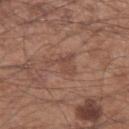Clinical impression:
Recorded during total-body skin imaging; not selected for excision or biopsy.
Context:
Automated tile analysis of the lesion measured roughly 7 lightness units darker than nearby skin and a normalized lesion–skin contrast near 5. The analysis additionally found a nevus-likeness score of about 0/100 and lesion-presence confidence of about 90/100. Imaged with white-light lighting. The lesion is located on the left forearm. A 15 mm close-up extracted from a 3D total-body photography capture. A male subject, aged around 55. Approximately 3.5 mm at its widest.